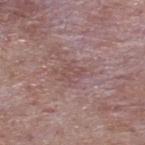follow-up: imaged on a skin check; not biopsied
patient: male, aged 63 to 67
automated lesion analysis: an average lesion color of about L≈49 a*≈19 b*≈20 (CIELAB), a lesion–skin lightness drop of about 6, and a normalized border contrast of about 4.5; a border-irregularity rating of about 3.5/10, a color-variation rating of about 1.5/10, and a peripheral color-asymmetry measure near 0.5; an automated nevus-likeness rating near 0 out of 100 and a lesion-detection confidence of about 65/100
acquisition: ~15 mm crop, total-body skin-cancer survey
anatomic site: the upper back